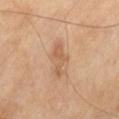This lesion was catalogued during total-body skin photography and was not selected for biopsy. The patient is a male approximately 70 years of age. This image is a 15 mm lesion crop taken from a total-body photograph. From the left thigh. Imaged with cross-polarized lighting.